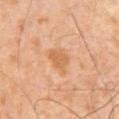{
  "biopsy_status": "not biopsied; imaged during a skin examination",
  "patient": {
    "sex": "male",
    "age_approx": 65
  },
  "image": {
    "source": "total-body photography crop",
    "field_of_view_mm": 15
  },
  "lesion_size": {
    "long_diameter_mm_approx": 3.0
  }
}